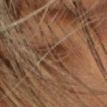This lesion was catalogued during total-body skin photography and was not selected for biopsy.
The lesion is located on the head or neck.
A 15 mm close-up tile from a total-body photography series done for melanoma screening.
The subject is a female in their mid-50s.